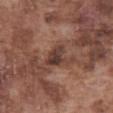Clinical impression:
The lesion was tiled from a total-body skin photograph and was not biopsied.
Background:
From the abdomen. A roughly 15 mm field-of-view crop from a total-body skin photograph. A male patient aged around 75.